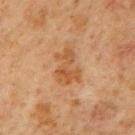An algorithmic analysis of the crop reported an area of roughly 8 mm² and a shape eccentricity near 0.8. The analysis additionally found an average lesion color of about L≈46 a*≈20 b*≈35 (CIELAB), a lesion–skin lightness drop of about 8, and a normalized border contrast of about 7. The software also gave a border-irregularity index near 7/10, internal color variation of about 3 on a 0–10 scale, and a peripheral color-asymmetry measure near 1. The analysis additionally found a classifier nevus-likeness of about 0/100 and a lesion-detection confidence of about 100/100.
A male subject, aged approximately 65.
Measured at roughly 4 mm in maximum diameter.
This is a cross-polarized tile.
A 15 mm close-up tile from a total-body photography series done for melanoma screening.
Located on the mid back.